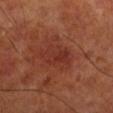<case>
  <site>right lower leg</site>
  <patient>
    <sex>male</sex>
    <age_approx>70</age_approx>
  </patient>
  <image>
    <source>total-body photography crop</source>
    <field_of_view_mm>15</field_of_view_mm>
  </image>
  <lesion_size>
    <long_diameter_mm_approx>5.0</long_diameter_mm_approx>
  </lesion_size>
</case>